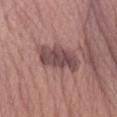This lesion was catalogued during total-body skin photography and was not selected for biopsy.
The patient is a male aged approximately 75.
A region of skin cropped from a whole-body photographic capture, roughly 15 mm wide.
On the left forearm.
Captured under white-light illumination.